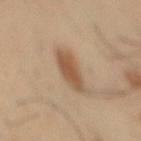{
  "biopsy_status": "not biopsied; imaged during a skin examination",
  "automated_metrics": {
    "nevus_likeness_0_100": 100,
    "lesion_detection_confidence_0_100": 100
  },
  "site": "lower back",
  "image": {
    "source": "total-body photography crop",
    "field_of_view_mm": 15
  },
  "lesion_size": {
    "long_diameter_mm_approx": 4.0
  },
  "patient": {
    "sex": "male",
    "age_approx": 40
  }
}